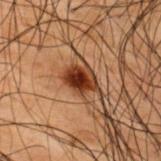Clinical impression: Recorded during total-body skin imaging; not selected for excision or biopsy. Clinical summary: The tile uses cross-polarized illumination. Automated image analysis of the tile measured a shape eccentricity near 0.8 and a shape-asymmetry score of about 0.3 (0 = symmetric). Cropped from a whole-body photographic skin survey; the tile spans about 15 mm. On the upper back. A male patient, about 50 years old. The lesion's longest dimension is about 3.5 mm.